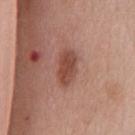biopsy_status: not biopsied; imaged during a skin examination
lesion_size:
  long_diameter_mm_approx: 4.0
image:
  source: total-body photography crop
  field_of_view_mm: 15
patient:
  sex: female
  age_approx: 65
lighting: white-light
site: front of the torso